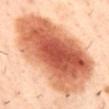A 15 mm close-up extracted from a 3D total-body photography capture. The lesion is located on the mid back. The tile uses cross-polarized illumination. About 11.5 mm across. The subject is a male about 40 years old.